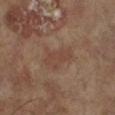Clinical impression: Captured during whole-body skin photography for melanoma surveillance; the lesion was not biopsied. Image and clinical context: Located on the right lower leg. Cropped from a total-body skin-imaging series; the visible field is about 15 mm. A female subject aged around 75. Measured at roughly 4 mm in maximum diameter. An algorithmic analysis of the crop reported a lesion area of about 8 mm² and a symmetry-axis asymmetry near 0.4. The software also gave a border-irregularity index near 4.5/10 and a color-variation rating of about 2/10.